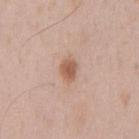Case summary:
* site · the front of the torso
* tile lighting · white-light
* subject · male, aged 48 to 52
* size · ≈3 mm
* automated metrics · a footprint of about 4.5 mm², an eccentricity of roughly 0.7, and a symmetry-axis asymmetry near 0.2
* image source · ~15 mm tile from a whole-body skin photo